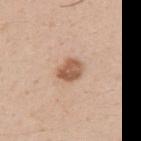No biopsy was performed on this lesion — it was imaged during a full skin examination and was not determined to be concerning.